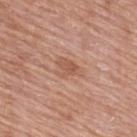Impression:
This lesion was catalogued during total-body skin photography and was not selected for biopsy.
Acquisition and patient details:
A close-up tile cropped from a whole-body skin photograph, about 15 mm across. Approximately 3.5 mm at its widest. A female subject aged 58–62. Automated tile analysis of the lesion measured a lesion area of about 5 mm², a shape eccentricity near 0.75, and a shape-asymmetry score of about 0.45 (0 = symmetric). The analysis additionally found a lesion color around L≈55 a*≈23 b*≈31 in CIELAB and roughly 8 lightness units darker than nearby skin. It also reported border irregularity of about 4.5 on a 0–10 scale, a within-lesion color-variation index near 2/10, and radial color variation of about 0.5. The tile uses white-light illumination. The lesion is located on the upper back.